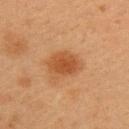notes: catalogued during a skin exam; not biopsied
size: ~4 mm (longest diameter)
image-analysis metrics: an average lesion color of about L≈44 a*≈23 b*≈35 (CIELAB) and roughly 9 lightness units darker than nearby skin; a nevus-likeness score of about 100/100
imaging modality: total-body-photography crop, ~15 mm field of view
subject: female, approximately 40 years of age
lighting: cross-polarized
body site: the left upper arm